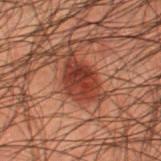Case summary:
– notes: catalogued during a skin exam; not biopsied
– patient: male, aged approximately 50
– lesion size: ~5.5 mm (longest diameter)
– image: 15 mm crop, total-body photography
– lighting: cross-polarized
– location: the leg